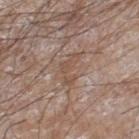Q: Is there a histopathology result?
A: imaged on a skin check; not biopsied
Q: How large is the lesion?
A: ~4 mm (longest diameter)
Q: What is the imaging modality?
A: total-body-photography crop, ~15 mm field of view
Q: What did automated image analysis measure?
A: a within-lesion color-variation index near 1.5/10 and peripheral color asymmetry of about 0; a nevus-likeness score of about 0/100 and lesion-presence confidence of about 60/100
Q: Lesion location?
A: the right lower leg
Q: Who is the patient?
A: male, aged around 60
Q: What lighting was used for the tile?
A: white-light